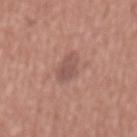Q: Is there a histopathology result?
A: imaged on a skin check; not biopsied
Q: Lesion location?
A: the abdomen
Q: What is the lesion's diameter?
A: about 3.5 mm
Q: What are the patient's age and sex?
A: male, about 45 years old
Q: What kind of image is this?
A: total-body-photography crop, ~15 mm field of view